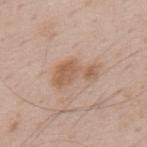  biopsy_status: not biopsied; imaged during a skin examination
  site: back
  lighting: white-light
  lesion_size:
    long_diameter_mm_approx: 5.5
  patient:
    sex: male
    age_approx: 70
  automated_metrics:
    cielab_L: 58
    cielab_a: 19
    cielab_b: 30
    vs_skin_contrast_norm: 7.0
    border_irregularity_0_10: 6.0
    color_variation_0_10: 2.5
    peripheral_color_asymmetry: 0.5
    nevus_likeness_0_100: 0
  image:
    source: total-body photography crop
    field_of_view_mm: 15